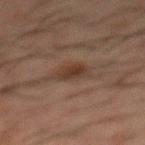No biopsy was performed on this lesion — it was imaged during a full skin examination and was not determined to be concerning.
Captured under cross-polarized illumination.
From the mid back.
Automated image analysis of the tile measured a lesion color around L≈28 a*≈13 b*≈20 in CIELAB and a normalized lesion–skin contrast near 7. The software also gave a border-irregularity rating of about 2.5/10, internal color variation of about 2.5 on a 0–10 scale, and peripheral color asymmetry of about 1. It also reported lesion-presence confidence of about 100/100.
A region of skin cropped from a whole-body photographic capture, roughly 15 mm wide.
Longest diameter approximately 3.5 mm.
The subject is a male approximately 50 years of age.